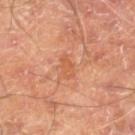The lesion is located on the leg. Measured at roughly 2.5 mm in maximum diameter. A 15 mm close-up extracted from a 3D total-body photography capture. The patient is a male aged approximately 70. Captured under cross-polarized illumination.